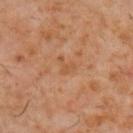<case>
  <biopsy_status>not biopsied; imaged during a skin examination</biopsy_status>
  <patient>
    <sex>male</sex>
    <age_approx>60</age_approx>
  </patient>
  <image>
    <source>total-body photography crop</source>
    <field_of_view_mm>15</field_of_view_mm>
  </image>
  <site>upper back</site>
  <automated_metrics>
    <area_mm2_approx>2.5</area_mm2_approx>
    <eccentricity>0.8</eccentricity>
    <shape_asymmetry>0.65</shape_asymmetry>
    <nevus_likeness_0_100>0</nevus_likeness_0_100>
    <lesion_detection_confidence_0_100>100</lesion_detection_confidence_0_100>
  </automated_metrics>
  <lesion_size>
    <long_diameter_mm_approx>2.5</long_diameter_mm_approx>
  </lesion_size>
  <lighting>cross-polarized</lighting>
</case>